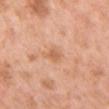  biopsy_status: not biopsied; imaged during a skin examination
  patient:
    sex: female
    age_approx: 50
  lighting: white-light
  image:
    source: total-body photography crop
    field_of_view_mm: 15
  site: chest